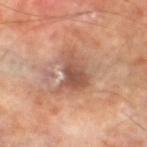notes: catalogued during a skin exam; not biopsied
illumination: cross-polarized
subject: male, approximately 70 years of age
acquisition: ~15 mm tile from a whole-body skin photo
anatomic site: the left lower leg
lesion diameter: about 5 mm